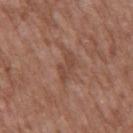Q: Was this lesion biopsied?
A: catalogued during a skin exam; not biopsied
Q: What are the patient's age and sex?
A: male, aged approximately 50
Q: Automated lesion metrics?
A: a footprint of about 4 mm² and two-axis asymmetry of about 0.4; a lesion–skin lightness drop of about 7 and a normalized lesion–skin contrast near 6
Q: Lesion size?
A: ≈3.5 mm
Q: Where on the body is the lesion?
A: the mid back
Q: What kind of image is this?
A: ~15 mm crop, total-body skin-cancer survey